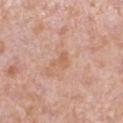Captured during whole-body skin photography for melanoma surveillance; the lesion was not biopsied. This image is a 15 mm lesion crop taken from a total-body photograph. A female subject, about 40 years old. Imaged with white-light lighting. The total-body-photography lesion software estimated an average lesion color of about L≈61 a*≈22 b*≈32 (CIELAB), about 6 CIELAB-L* units darker than the surrounding skin, and a lesion-to-skin contrast of about 5 (normalized; higher = more distinct). And it measured border irregularity of about 5 on a 0–10 scale, a within-lesion color-variation index near 2/10, and a peripheral color-asymmetry measure near 1. The software also gave a classifier nevus-likeness of about 0/100 and a detector confidence of about 100 out of 100 that the crop contains a lesion. The lesion is on the left lower leg.